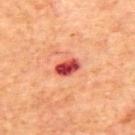Case summary:
• notes: total-body-photography surveillance lesion; no biopsy
• diameter: ≈3 mm
• tile lighting: cross-polarized
• image-analysis metrics: a footprint of about 5 mm², a shape eccentricity near 0.8, and a symmetry-axis asymmetry near 0.15; a lesion color around L≈50 a*≈42 b*≈33 in CIELAB, roughly 20 lightness units darker than nearby skin, and a normalized border contrast of about 13; internal color variation of about 5 on a 0–10 scale and peripheral color asymmetry of about 2
• site: the upper back
• subject: male, aged 68 to 72
• imaging modality: total-body-photography crop, ~15 mm field of view